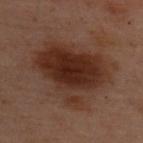This lesion was catalogued during total-body skin photography and was not selected for biopsy.
A 15 mm close-up tile from a total-body photography series done for melanoma screening.
Longest diameter approximately 8 mm.
A female patient aged 53–57.
The lesion is located on the upper back.
The total-body-photography lesion software estimated an area of roughly 34 mm², a shape eccentricity near 0.6, and a shape-asymmetry score of about 0.35 (0 = symmetric). The analysis additionally found border irregularity of about 5 on a 0–10 scale and internal color variation of about 3.5 on a 0–10 scale. It also reported a nevus-likeness score of about 95/100 and lesion-presence confidence of about 100/100.
Captured under cross-polarized illumination.